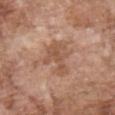Impression: The lesion was tiled from a total-body skin photograph and was not biopsied. Clinical summary: The total-body-photography lesion software estimated a lesion area of about 9.5 mm², an eccentricity of roughly 0.6, and two-axis asymmetry of about 0.5. A lesion tile, about 15 mm wide, cut from a 3D total-body photograph. This is a white-light tile. A male subject aged approximately 70. The lesion's longest dimension is about 4 mm. From the chest.